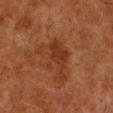This lesion was catalogued during total-body skin photography and was not selected for biopsy. Imaged with cross-polarized lighting. A male patient, about 80 years old. A close-up tile cropped from a whole-body skin photograph, about 15 mm across. The lesion-visualizer software estimated a footprint of about 11 mm², a shape eccentricity near 0.8, and a shape-asymmetry score of about 0.6 (0 = symmetric). And it measured a lesion color around L≈27 a*≈21 b*≈27 in CIELAB, roughly 6 lightness units darker than nearby skin, and a normalized lesion–skin contrast near 6. And it measured border irregularity of about 7 on a 0–10 scale, a within-lesion color-variation index near 2.5/10, and a peripheral color-asymmetry measure near 1. Measured at roughly 5.5 mm in maximum diameter. On the left lower leg.